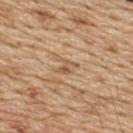The lesion was tiled from a total-body skin photograph and was not biopsied. A male subject, roughly 70 years of age. The total-body-photography lesion software estimated an area of roughly 4 mm² and an outline eccentricity of about 0.7 (0 = round, 1 = elongated). And it measured a nevus-likeness score of about 0/100 and a lesion-detection confidence of about 70/100. The tile uses white-light illumination. The lesion is located on the back. A 15 mm close-up extracted from a 3D total-body photography capture. Approximately 3 mm at its widest.